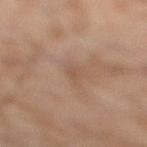biopsy_status: not biopsied; imaged during a skin examination
patient:
  sex: male
  age_approx: 55
site: leg
lighting: cross-polarized
image:
  source: total-body photography crop
  field_of_view_mm: 15
lesion_size:
  long_diameter_mm_approx: 2.5